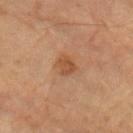Impression:
Recorded during total-body skin imaging; not selected for excision or biopsy.
Image and clinical context:
The total-body-photography lesion software estimated a mean CIELAB color near L≈43 a*≈20 b*≈31, about 7 CIELAB-L* units darker than the surrounding skin, and a lesion-to-skin contrast of about 6.5 (normalized; higher = more distinct). The lesion is located on the left forearm. Measured at roughly 2.5 mm in maximum diameter. A lesion tile, about 15 mm wide, cut from a 3D total-body photograph. A male patient about 85 years old or older. This is a cross-polarized tile.